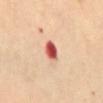The lesion was photographed on a routine skin check and not biopsied; there is no pathology result. The recorded lesion diameter is about 2.5 mm. This image is a 15 mm lesion crop taken from a total-body photograph. Located on the front of the torso. Captured under cross-polarized illumination. The subject is a female aged around 60.